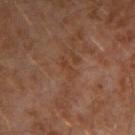Findings:
• biopsy status — total-body-photography surveillance lesion; no biopsy
• patient — male, aged 28–32
• imaging modality — total-body-photography crop, ~15 mm field of view
• anatomic site — the left arm